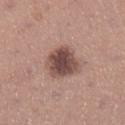Assessment:
Captured during whole-body skin photography for melanoma surveillance; the lesion was not biopsied.
Background:
The recorded lesion diameter is about 4.5 mm. The tile uses white-light illumination. Automated tile analysis of the lesion measured a shape eccentricity near 0.55 and two-axis asymmetry of about 0.2. And it measured a mean CIELAB color near L≈48 a*≈19 b*≈22, a lesion–skin lightness drop of about 15, and a normalized lesion–skin contrast near 10.5. It also reported a classifier nevus-likeness of about 30/100 and a detector confidence of about 100 out of 100 that the crop contains a lesion. From the left lower leg. A female patient aged around 30. A 15 mm close-up extracted from a 3D total-body photography capture.